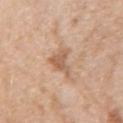lighting = white-light illumination
subject = female, aged approximately 70
anatomic site = the left upper arm
imaging modality = 15 mm crop, total-body photography